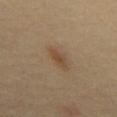A 15 mm close-up extracted from a 3D total-body photography capture. Automated tile analysis of the lesion measured two-axis asymmetry of about 0.35. The analysis additionally found a lesion color around L≈43 a*≈16 b*≈30 in CIELAB, roughly 8 lightness units darker than nearby skin, and a lesion-to-skin contrast of about 7 (normalized; higher = more distinct). It also reported border irregularity of about 3.5 on a 0–10 scale, a color-variation rating of about 1/10, and a peripheral color-asymmetry measure near 0.5. The software also gave an automated nevus-likeness rating near 90 out of 100 and a lesion-detection confidence of about 100/100. Captured under cross-polarized illumination. The lesion is located on the abdomen. The patient is a male aged 58 to 62.